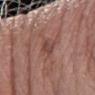Imaged during a routine full-body skin examination; the lesion was not biopsied and no histopathology is available. Automated tile analysis of the lesion measured a border-irregularity rating of about 3.5/10 and a color-variation rating of about 2/10. It also reported a classifier nevus-likeness of about 0/100 and a lesion-detection confidence of about 90/100. The lesion is located on the left forearm. A close-up tile cropped from a whole-body skin photograph, about 15 mm across. The recorded lesion diameter is about 3 mm. Imaged with white-light lighting. The patient is a female aged approximately 60.